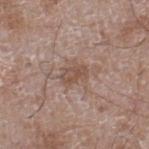The lesion was tiled from a total-body skin photograph and was not biopsied. The recorded lesion diameter is about 3 mm. A male patient, aged approximately 60. A roughly 15 mm field-of-view crop from a total-body skin photograph. From the right lower leg. The lesion-visualizer software estimated a footprint of about 5 mm², an eccentricity of roughly 0.75, and two-axis asymmetry of about 0.25. And it measured a lesion color around L≈50 a*≈17 b*≈25 in CIELAB, roughly 8 lightness units darker than nearby skin, and a lesion-to-skin contrast of about 6 (normalized; higher = more distinct).